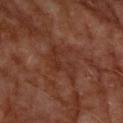Clinical impression: This lesion was catalogued during total-body skin photography and was not selected for biopsy. Context: From the left upper arm. A roughly 15 mm field-of-view crop from a total-body skin photograph. About 5.5 mm across. A male patient, roughly 60 years of age. Imaged with cross-polarized lighting.